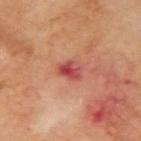This lesion was catalogued during total-body skin photography and was not selected for biopsy. The lesion is located on the right upper arm. A male subject about 70 years old. A 15 mm close-up tile from a total-body photography series done for melanoma screening. This is a cross-polarized tile.